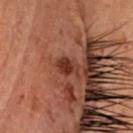illumination = cross-polarized illumination; patient = male, aged 38–42; diameter = ≈2.5 mm; acquisition = ~15 mm tile from a whole-body skin photo; image-analysis metrics = a lesion color around L≈27 a*≈22 b*≈24 in CIELAB, about 8 CIELAB-L* units darker than the surrounding skin, and a normalized lesion–skin contrast near 8.5; site = the head or neck.